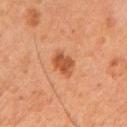follow-up: total-body-photography surveillance lesion; no biopsy | imaging modality: ~15 mm crop, total-body skin-cancer survey | tile lighting: cross-polarized illumination | subject: female, roughly 60 years of age | automated metrics: an area of roughly 5.5 mm² and a symmetry-axis asymmetry near 0.2; a mean CIELAB color near L≈42 a*≈24 b*≈33 and a lesion-to-skin contrast of about 8 (normalized; higher = more distinct); a border-irregularity rating of about 2.5/10, internal color variation of about 2 on a 0–10 scale, and radial color variation of about 0.5; an automated nevus-likeness rating near 90 out of 100 and a lesion-detection confidence of about 100/100 | lesion size: ≈3.5 mm | site: the right forearm.